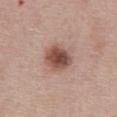{
  "biopsy_status": "not biopsied; imaged during a skin examination",
  "automated_metrics": {
    "vs_skin_darker_L": 15.0,
    "vs_skin_contrast_norm": 10.0
  },
  "site": "abdomen",
  "image": {
    "source": "total-body photography crop",
    "field_of_view_mm": 15
  },
  "lighting": "white-light",
  "lesion_size": {
    "long_diameter_mm_approx": 3.5
  },
  "patient": {
    "sex": "male",
    "age_approx": 60
  }
}